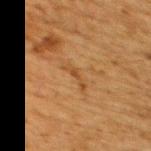This lesion was catalogued during total-body skin photography and was not selected for biopsy. The subject is a male approximately 60 years of age. A roughly 15 mm field-of-view crop from a total-body skin photograph. Measured at roughly 3 mm in maximum diameter. The lesion is on the back. Imaged with cross-polarized lighting.